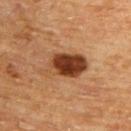The lesion was photographed on a routine skin check and not biopsied; there is no pathology result.
A male subject, aged 63–67.
A region of skin cropped from a whole-body photographic capture, roughly 15 mm wide.
The lesion is located on the upper back.
The tile uses cross-polarized illumination.
Automated tile analysis of the lesion measured a footprint of about 14 mm², a shape eccentricity near 0.8, and two-axis asymmetry of about 0.25. And it measured a mean CIELAB color near L≈37 a*≈22 b*≈32, a lesion–skin lightness drop of about 15, and a normalized border contrast of about 12. The software also gave border irregularity of about 3.5 on a 0–10 scale and internal color variation of about 8 on a 0–10 scale. It also reported an automated nevus-likeness rating near 95 out of 100.